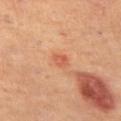biopsy_status: not biopsied; imaged during a skin examination
lighting: cross-polarized
site: left thigh
lesion_size:
  long_diameter_mm_approx: 2.0
image:
  source: total-body photography crop
  field_of_view_mm: 15
patient:
  sex: female
  age_approx: 40